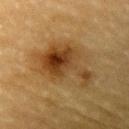  biopsy_status: not biopsied; imaged during a skin examination
  image:
    source: total-body photography crop
    field_of_view_mm: 15
  lesion_size:
    long_diameter_mm_approx: 7.0
  patient:
    sex: male
    age_approx: 85+
  site: left upper arm
  lighting: cross-polarized